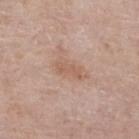Notes:
- workup — no biopsy performed (imaged during a skin exam)
- tile lighting — white-light illumination
- patient — male, aged around 80
- lesion size — about 3.5 mm
- acquisition — ~15 mm crop, total-body skin-cancer survey
- automated metrics — a footprint of about 3.5 mm² and a shape eccentricity near 0.9; a lesion color around L≈58 a*≈19 b*≈29 in CIELAB and a lesion-to-skin contrast of about 5.5 (normalized; higher = more distinct); a border-irregularity rating of about 4.5/10, internal color variation of about 0.5 on a 0–10 scale, and radial color variation of about 0; a nevus-likeness score of about 0/100
- anatomic site — the right lower leg